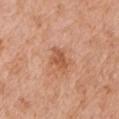Captured during whole-body skin photography for melanoma surveillance; the lesion was not biopsied.
Automated tile analysis of the lesion measured an area of roughly 5 mm², an eccentricity of roughly 0.75, and a symmetry-axis asymmetry near 0.35. And it measured about 10 CIELAB-L* units darker than the surrounding skin and a normalized lesion–skin contrast near 7.
From the left upper arm.
The subject is a male aged around 60.
A lesion tile, about 15 mm wide, cut from a 3D total-body photograph.
Captured under white-light illumination.
Measured at roughly 3 mm in maximum diameter.